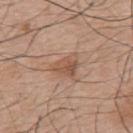Impression:
Part of a total-body skin-imaging series; this lesion was reviewed on a skin check and was not flagged for biopsy.
Context:
The tile uses white-light illumination. On the mid back. A region of skin cropped from a whole-body photographic capture, roughly 15 mm wide. A male subject, in their mid-70s. An algorithmic analysis of the crop reported a lesion color around L≈53 a*≈20 b*≈29 in CIELAB and a lesion–skin lightness drop of about 9. And it measured a detector confidence of about 100 out of 100 that the crop contains a lesion.